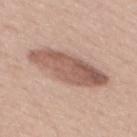<record>
  <biopsy_status>not biopsied; imaged during a skin examination</biopsy_status>
  <automated_metrics>
    <vs_skin_darker_L>13.0</vs_skin_darker_L>
    <vs_skin_contrast_norm>8.5</vs_skin_contrast_norm>
    <border_irregularity_0_10>2.5</border_irregularity_0_10>
    <nevus_likeness_0_100>35</nevus_likeness_0_100>
  </automated_metrics>
  <site>mid back</site>
  <image>
    <source>total-body photography crop</source>
    <field_of_view_mm>15</field_of_view_mm>
  </image>
  <lighting>white-light</lighting>
  <lesion_size>
    <long_diameter_mm_approx>8.0</long_diameter_mm_approx>
  </lesion_size>
  <patient>
    <sex>female</sex>
    <age_approx>55</age_approx>
  </patient>
</record>